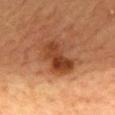| feature | finding |
|---|---|
| workup | catalogued during a skin exam; not biopsied |
| anatomic site | the mid back |
| illumination | cross-polarized |
| image | total-body-photography crop, ~15 mm field of view |
| patient | female, roughly 60 years of age |